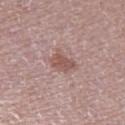Findings:
* follow-up — catalogued during a skin exam; not biopsied
* imaging modality — total-body-photography crop, ~15 mm field of view
* automated lesion analysis — a lesion color around L≈53 a*≈21 b*≈22 in CIELAB and a normalized lesion–skin contrast near 7; border irregularity of about 3.5 on a 0–10 scale and a peripheral color-asymmetry measure near 0.5; a nevus-likeness score of about 55/100 and a detector confidence of about 100 out of 100 that the crop contains a lesion
* subject — male, in their mid-70s
* tile lighting — white-light illumination
* size — ~3 mm (longest diameter)
* anatomic site — the right thigh